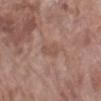notes: no biopsy performed (imaged during a skin exam)
lesion size: about 2.5 mm
image: ~15 mm tile from a whole-body skin photo
location: the left forearm
lighting: white-light illumination
subject: female, approximately 70 years of age